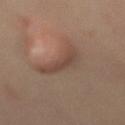Impression: The lesion was photographed on a routine skin check and not biopsied; there is no pathology result. Context: A roughly 15 mm field-of-view crop from a total-body skin photograph. The lesion is on the back. About 5 mm across. The tile uses cross-polarized illumination. A female patient, in their mid- to late 40s.